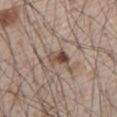No biopsy was performed on this lesion — it was imaged during a full skin examination and was not determined to be concerning.
The tile uses white-light illumination.
The lesion is located on the chest.
The total-body-photography lesion software estimated a footprint of about 4.5 mm², a shape eccentricity near 0.75, and two-axis asymmetry of about 0.25. The analysis additionally found a lesion-to-skin contrast of about 9 (normalized; higher = more distinct). It also reported a border-irregularity rating of about 2.5/10, internal color variation of about 9 on a 0–10 scale, and a peripheral color-asymmetry measure near 4. The software also gave a nevus-likeness score of about 90/100 and lesion-presence confidence of about 100/100.
Cropped from a whole-body photographic skin survey; the tile spans about 15 mm.
About 2.5 mm across.
A male patient in their mid- to late 60s.